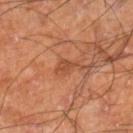Case summary:
* biopsy status: catalogued during a skin exam; not biopsied
* patient: male, roughly 60 years of age
* TBP lesion metrics: an eccentricity of roughly 0.8 and a symmetry-axis asymmetry near 0.25; a border-irregularity index near 2.5/10, a within-lesion color-variation index near 1.5/10, and a peripheral color-asymmetry measure near 0.5
* image source: 15 mm crop, total-body photography
* lighting: cross-polarized illumination
* lesion size: ~2.5 mm (longest diameter)
* location: the right lower leg